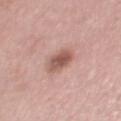follow-up: catalogued during a skin exam; not biopsied
subject: female, in their mid- to late 50s
tile lighting: white-light illumination
imaging modality: total-body-photography crop, ~15 mm field of view
site: the right thigh